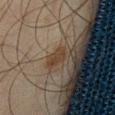follow-up — no biopsy performed (imaged during a skin exam) | acquisition — ~15 mm crop, total-body skin-cancer survey | illumination — cross-polarized illumination | anatomic site — the left thigh | diameter — ~2.5 mm (longest diameter) | subject — male, aged approximately 45 | automated lesion analysis — an area of roughly 4 mm² and a symmetry-axis asymmetry near 0.3; a mean CIELAB color near L≈31 a*≈12 b*≈23, about 5 CIELAB-L* units darker than the surrounding skin, and a lesion-to-skin contrast of about 7.5 (normalized; higher = more distinct); a classifier nevus-likeness of about 85/100 and lesion-presence confidence of about 100/100.